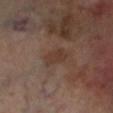The lesion was photographed on a routine skin check and not biopsied; there is no pathology result. A male patient, aged 68–72. Located on the left lower leg. Cropped from a whole-body photographic skin survey; the tile spans about 15 mm. Captured under cross-polarized illumination. About 3.5 mm across.